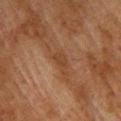Q: Was this lesion biopsied?
A: imaged on a skin check; not biopsied
Q: Who is the patient?
A: male, aged around 75
Q: Lesion location?
A: the upper back
Q: What kind of image is this?
A: 15 mm crop, total-body photography
Q: Illumination type?
A: cross-polarized